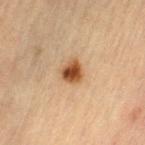Q: Was this lesion biopsied?
A: total-body-photography surveillance lesion; no biopsy
Q: What is the imaging modality?
A: 15 mm crop, total-body photography
Q: Lesion location?
A: the left thigh
Q: What are the patient's age and sex?
A: female, aged approximately 65
Q: Illumination type?
A: cross-polarized
Q: How large is the lesion?
A: ~2.5 mm (longest diameter)